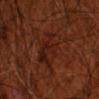| key | value |
|---|---|
| biopsy status | catalogued during a skin exam; not biopsied |
| patient | male, in their 70s |
| body site | the arm |
| illumination | cross-polarized |
| image | 15 mm crop, total-body photography |
| image-analysis metrics | an eccentricity of roughly 0.85 and a symmetry-axis asymmetry near 0.35; an average lesion color of about L≈21 a*≈22 b*≈25 (CIELAB), about 5 CIELAB-L* units darker than the surrounding skin, and a lesion-to-skin contrast of about 6.5 (normalized; higher = more distinct); a border-irregularity rating of about 5/10, a within-lesion color-variation index near 5/10, and a peripheral color-asymmetry measure near 2; an automated nevus-likeness rating near 5 out of 100 and a detector confidence of about 90 out of 100 that the crop contains a lesion |
| lesion size | about 6.5 mm |